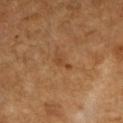Part of a total-body skin-imaging series; this lesion was reviewed on a skin check and was not flagged for biopsy. On the left forearm. A 15 mm close-up extracted from a 3D total-body photography capture. The patient is a female in their 60s.